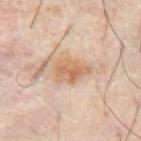biopsy status = no biopsy performed (imaged during a skin exam) | imaging modality = 15 mm crop, total-body photography | subject = male, aged 58 to 62 | automated metrics = a footprint of about 9.5 mm² and two-axis asymmetry of about 0.3; about 9 CIELAB-L* units darker than the surrounding skin and a normalized lesion–skin contrast near 7; border irregularity of about 3.5 on a 0–10 scale and radial color variation of about 1.5 | body site = the abdomen | lesion diameter = about 4 mm.